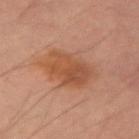Background:
The patient is a male in their mid-30s. On the right upper arm. This image is a 15 mm lesion crop taken from a total-body photograph.